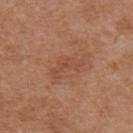The subject is a female roughly 40 years of age. Captured under white-light illumination. The recorded lesion diameter is about 4.5 mm. Automated tile analysis of the lesion measured an average lesion color of about L≈48 a*≈23 b*≈31 (CIELAB), a lesion–skin lightness drop of about 6, and a lesion-to-skin contrast of about 5 (normalized; higher = more distinct). The analysis additionally found a within-lesion color-variation index near 1/10 and peripheral color asymmetry of about 0. It also reported an automated nevus-likeness rating near 0 out of 100 and lesion-presence confidence of about 100/100. Located on the mid back. A lesion tile, about 15 mm wide, cut from a 3D total-body photograph.